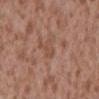The subject is a male aged approximately 45.
A close-up tile cropped from a whole-body skin photograph, about 15 mm across.
Approximately 2.5 mm at its widest.
Located on the mid back.
Captured under white-light illumination.